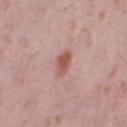{"biopsy_status": "not biopsied; imaged during a skin examination", "lighting": "white-light", "image": {"source": "total-body photography crop", "field_of_view_mm": 15}, "lesion_size": {"long_diameter_mm_approx": 3.0}, "patient": {"sex": "female", "age_approx": 40}, "site": "right thigh", "automated_metrics": {"eccentricity": 0.75, "cielab_L": 54, "cielab_a": 25, "cielab_b": 24, "vs_skin_darker_L": 11.0, "nevus_likeness_0_100": 60, "lesion_detection_confidence_0_100": 100}}